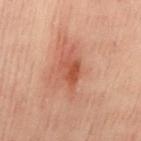  biopsy_status: not biopsied; imaged during a skin examination
  image:
    source: total-body photography crop
    field_of_view_mm: 15
  automated_metrics:
    area_mm2_approx: 6.5
    eccentricity: 0.7
    shape_asymmetry: 0.3
    border_irregularity_0_10: 3.0
    color_variation_0_10: 5.0
    nevus_likeness_0_100: 5
  lighting: cross-polarized
  site: back
  patient:
    sex: male
    age_approx: 40